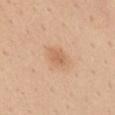Assessment:
Captured during whole-body skin photography for melanoma surveillance; the lesion was not biopsied.
Acquisition and patient details:
Located on the mid back. A female patient, approximately 40 years of age. Longest diameter approximately 2.5 mm. Imaged with white-light lighting. A region of skin cropped from a whole-body photographic capture, roughly 15 mm wide. An algorithmic analysis of the crop reported a border-irregularity rating of about 2/10, internal color variation of about 1.5 on a 0–10 scale, and a peripheral color-asymmetry measure near 0.5. And it measured a classifier nevus-likeness of about 35/100 and lesion-presence confidence of about 100/100.